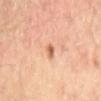| key | value |
|---|---|
| subject | male, aged around 55 |
| size | ≈2 mm |
| illumination | cross-polarized illumination |
| anatomic site | the mid back |
| acquisition | 15 mm crop, total-body photography |